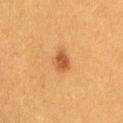Impression: No biopsy was performed on this lesion — it was imaged during a full skin examination and was not determined to be concerning.